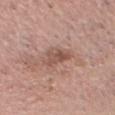Context:
A male patient about 60 years old. The lesion is located on the head or neck. Cropped from a total-body skin-imaging series; the visible field is about 15 mm.